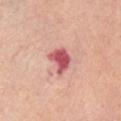follow-up: no biopsy performed (imaged during a skin exam) | patient: female, about 70 years old | anatomic site: the abdomen | imaging modality: ~15 mm crop, total-body skin-cancer survey.